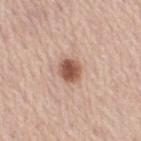Captured during whole-body skin photography for melanoma surveillance; the lesion was not biopsied. A female patient, approximately 65 years of age. This image is a 15 mm lesion crop taken from a total-body photograph. Located on the right thigh. This is a white-light tile. Measured at roughly 2.5 mm in maximum diameter.